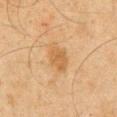Clinical impression: The lesion was tiled from a total-body skin photograph and was not biopsied. Background: Captured under cross-polarized illumination. A roughly 15 mm field-of-view crop from a total-body skin photograph. The lesion-visualizer software estimated an average lesion color of about L≈49 a*≈17 b*≈36 (CIELAB), roughly 7 lightness units darker than nearby skin, and a lesion-to-skin contrast of about 6.5 (normalized; higher = more distinct). A male patient, aged 63 to 67. Located on the arm.